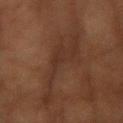Findings:
- notes: no biopsy performed (imaged during a skin exam)
- lighting: cross-polarized illumination
- body site: the right forearm
- imaging modality: ~15 mm tile from a whole-body skin photo
- image-analysis metrics: an average lesion color of about L≈23 a*≈14 b*≈20 (CIELAB) and roughly 4 lightness units darker than nearby skin; a classifier nevus-likeness of about 0/100
- patient: female, aged 78 to 82